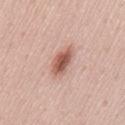Notes:
- biopsy status — no biopsy performed (imaged during a skin exam)
- anatomic site — the back
- subject — male, roughly 55 years of age
- size — ~4 mm (longest diameter)
- image-analysis metrics — a shape eccentricity near 0.8 and a shape-asymmetry score of about 0.2 (0 = symmetric); about 14 CIELAB-L* units darker than the surrounding skin and a normalized border contrast of about 9; a border-irregularity rating of about 2/10, a within-lesion color-variation index near 4.5/10, and a peripheral color-asymmetry measure near 1; an automated nevus-likeness rating near 95 out of 100 and a lesion-detection confidence of about 100/100
- illumination — white-light
- acquisition — ~15 mm tile from a whole-body skin photo